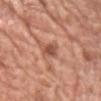Captured during whole-body skin photography for melanoma surveillance; the lesion was not biopsied. A male subject aged 78 to 82. A close-up tile cropped from a whole-body skin photograph, about 15 mm across. Captured under white-light illumination. Approximately 3 mm at its widest. Located on the chest.